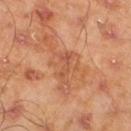The lesion was tiled from a total-body skin photograph and was not biopsied.
The total-body-photography lesion software estimated a border-irregularity rating of about 7/10. And it measured an automated nevus-likeness rating near 0 out of 100 and a detector confidence of about 100 out of 100 that the crop contains a lesion.
The lesion is located on the left lower leg.
A lesion tile, about 15 mm wide, cut from a 3D total-body photograph.
The subject is a male in their mid-40s.
The lesion's longest dimension is about 3 mm.
Imaged with cross-polarized lighting.